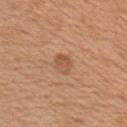  site: chest
  patient:
    sex: male
    age_approx: 65
  image:
    source: total-body photography crop
    field_of_view_mm: 15